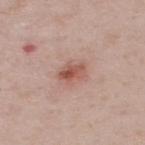No biopsy was performed on this lesion — it was imaged during a full skin examination and was not determined to be concerning. The lesion's longest dimension is about 3 mm. This is a white-light tile. A region of skin cropped from a whole-body photographic capture, roughly 15 mm wide. On the upper back. Automated tile analysis of the lesion measured an area of roughly 4.5 mm², an eccentricity of roughly 0.8, and two-axis asymmetry of about 0.2. And it measured internal color variation of about 5.5 on a 0–10 scale and peripheral color asymmetry of about 2. The subject is a male approximately 50 years of age.